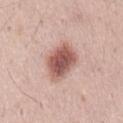Findings:
– notes — catalogued during a skin exam; not biopsied
– tile lighting — white-light illumination
– imaging modality — total-body-photography crop, ~15 mm field of view
– image-analysis metrics — an area of roughly 13 mm² and a shape eccentricity near 0.75; a mean CIELAB color near L≈56 a*≈23 b*≈24, a lesion–skin lightness drop of about 16, and a lesion-to-skin contrast of about 10.5 (normalized; higher = more distinct)
– patient — male, aged around 35
– site — the mid back